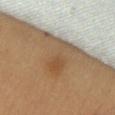Notes:
* workup · imaged on a skin check; not biopsied
* image source · ~15 mm crop, total-body skin-cancer survey
* lesion diameter · ≈7.5 mm
* location · the chest
* subject · female, aged 58–62